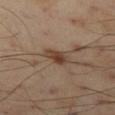biopsy_status: not biopsied; imaged during a skin examination
lesion_size:
  long_diameter_mm_approx: 3.5
site: left thigh
image:
  source: total-body photography crop
  field_of_view_mm: 15
lighting: cross-polarized
patient:
  sex: male
  age_approx: 55
automated_metrics:
  area_mm2_approx: 4.5
  eccentricity: 0.85
  shape_asymmetry: 0.25
  cielab_L: 41
  cielab_a: 17
  cielab_b: 27
  vs_skin_contrast_norm: 9.0
  border_irregularity_0_10: 2.5
  color_variation_0_10: 4.0
  nevus_likeness_0_100: 75
  lesion_detection_confidence_0_100: 100